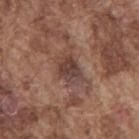Part of a total-body skin-imaging series; this lesion was reviewed on a skin check and was not flagged for biopsy. A male subject aged 73–77. Located on the mid back. The lesion-visualizer software estimated a lesion area of about 5.5 mm², a shape eccentricity near 0.6, and a shape-asymmetry score of about 0.3 (0 = symmetric). The software also gave a within-lesion color-variation index near 4/10 and a peripheral color-asymmetry measure near 1.5. The software also gave an automated nevus-likeness rating near 0 out of 100 and lesion-presence confidence of about 90/100. A lesion tile, about 15 mm wide, cut from a 3D total-body photograph. This is a white-light tile. Approximately 3 mm at its widest.